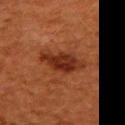This image is a 15 mm lesion crop taken from a total-body photograph.
An algorithmic analysis of the crop reported a shape eccentricity near 0.85 and a symmetry-axis asymmetry near 0.2. And it measured internal color variation of about 4 on a 0–10 scale. The software also gave a nevus-likeness score of about 80/100 and lesion-presence confidence of about 100/100.
A female subject, aged 48–52.
From the upper back.
This is a cross-polarized tile.